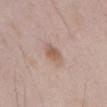- follow-up — catalogued during a skin exam; not biopsied
- lighting — white-light
- patient — male, aged around 55
- diameter — ≈3 mm
- image source — 15 mm crop, total-body photography
- anatomic site — the abdomen
- automated lesion analysis — a symmetry-axis asymmetry near 0.2; a mean CIELAB color near L≈58 a*≈17 b*≈28, about 9 CIELAB-L* units darker than the surrounding skin, and a lesion-to-skin contrast of about 7 (normalized; higher = more distinct); a nevus-likeness score of about 55/100 and a detector confidence of about 100 out of 100 that the crop contains a lesion